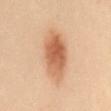lighting: cross-polarized; site: the mid back; image: total-body-photography crop, ~15 mm field of view; patient: female, roughly 60 years of age.